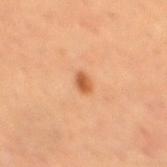Recorded during total-body skin imaging; not selected for excision or biopsy.